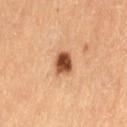– biopsy status — catalogued during a skin exam; not biopsied
– TBP lesion metrics — a footprint of about 5.5 mm² and an outline eccentricity of about 0.5 (0 = round, 1 = elongated); a classifier nevus-likeness of about 100/100
– image — ~15 mm tile from a whole-body skin photo
– location — the left thigh
– lighting — cross-polarized
– subject — female, in their 60s